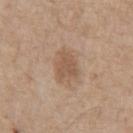{"biopsy_status": "not biopsied; imaged during a skin examination", "image": {"source": "total-body photography crop", "field_of_view_mm": 15}, "site": "chest", "automated_metrics": {"lesion_detection_confidence_0_100": 100}, "patient": {"sex": "male", "age_approx": 70}}